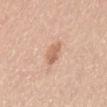<lesion>
  <patient>
    <sex>female</sex>
    <age_approx>55</age_approx>
  </patient>
  <lesion_size>
    <long_diameter_mm_approx>2.5</long_diameter_mm_approx>
  </lesion_size>
  <lighting>white-light</lighting>
  <site>back</site>
  <automated_metrics>
    <border_irregularity_0_10>3.0</border_irregularity_0_10>
    <color_variation_0_10>2.0</color_variation_0_10>
    <peripheral_color_asymmetry>0.5</peripheral_color_asymmetry>
  </automated_metrics>
  <image>
    <source>total-body photography crop</source>
    <field_of_view_mm>15</field_of_view_mm>
  </image>
</lesion>